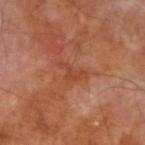biopsy status: no biopsy performed (imaged during a skin exam); diameter: ~3.5 mm (longest diameter); image: 15 mm crop, total-body photography; body site: the right forearm; subject: male, roughly 70 years of age.